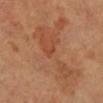The lesion was tiled from a total-body skin photograph and was not biopsied.
A 15 mm close-up extracted from a 3D total-body photography capture.
The lesion's longest dimension is about 1.5 mm.
This is a cross-polarized tile.
From the left lower leg.
A female patient aged 53 to 57.